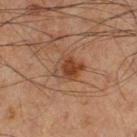Case summary:
- biopsy status · catalogued during a skin exam; not biopsied
- image · ~15 mm tile from a whole-body skin photo
- subject · male, in their 60s
- body site · the left thigh